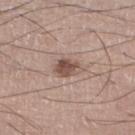follow-up — catalogued during a skin exam; not biopsied
imaging modality — total-body-photography crop, ~15 mm field of view
lighting — white-light illumination
patient — male, in their mid- to late 70s
lesion size — about 3 mm
body site — the leg